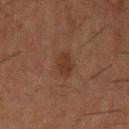Captured during whole-body skin photography for melanoma surveillance; the lesion was not biopsied. This image is a 15 mm lesion crop taken from a total-body photograph. The lesion-visualizer software estimated an average lesion color of about L≈26 a*≈17 b*≈23 (CIELAB), a lesion–skin lightness drop of about 5, and a lesion-to-skin contrast of about 6.5 (normalized; higher = more distinct). On the left upper arm. A male patient approximately 50 years of age. Longest diameter approximately 2.5 mm. The tile uses cross-polarized illumination.